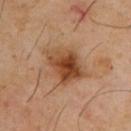{
  "biopsy_status": "not biopsied; imaged during a skin examination",
  "lesion_size": {
    "long_diameter_mm_approx": 5.0
  },
  "site": "upper back",
  "lighting": "cross-polarized",
  "automated_metrics": {
    "eccentricity": 0.7,
    "cielab_L": 43,
    "cielab_a": 21,
    "cielab_b": 32,
    "vs_skin_contrast_norm": 9.5
  },
  "patient": {
    "sex": "male",
    "age_approx": 65
  },
  "image": {
    "source": "total-body photography crop",
    "field_of_view_mm": 15
  }
}